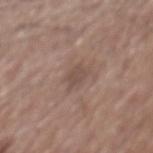The lesion was photographed on a routine skin check and not biopsied; there is no pathology result. Automated image analysis of the tile measured a mean CIELAB color near L≈48 a*≈16 b*≈22, roughly 7 lightness units darker than nearby skin, and a lesion-to-skin contrast of about 5.5 (normalized; higher = more distinct). And it measured a classifier nevus-likeness of about 0/100. The subject is a male aged 73 to 77. The tile uses white-light illumination. Measured at roughly 2.5 mm in maximum diameter. The lesion is on the mid back. A region of skin cropped from a whole-body photographic capture, roughly 15 mm wide.